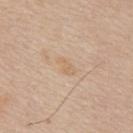The lesion was tiled from a total-body skin photograph and was not biopsied. On the back. A male subject about 65 years old. Cropped from a whole-body photographic skin survey; the tile spans about 15 mm. An algorithmic analysis of the crop reported an outline eccentricity of about 0.9 (0 = round, 1 = elongated) and two-axis asymmetry of about 0.4. The recorded lesion diameter is about 2.5 mm.